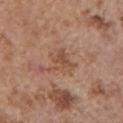Assessment:
The lesion was tiled from a total-body skin photograph and was not biopsied.
Image and clinical context:
A female subject, aged 63 to 67. The lesion is on the left upper arm. A close-up tile cropped from a whole-body skin photograph, about 15 mm across.